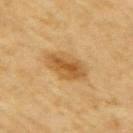tile lighting = cross-polarized illumination
diameter = ≈5 mm
location = the right upper arm
patient = female, aged 68 to 72
imaging modality = total-body-photography crop, ~15 mm field of view
TBP lesion metrics = a footprint of about 12 mm², an eccentricity of roughly 0.75, and two-axis asymmetry of about 0.2; a normalized border contrast of about 7.5; a nevus-likeness score of about 90/100